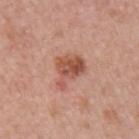follow-up — no biopsy performed (imaged during a skin exam) | anatomic site — the right upper arm | lesion diameter — ≈4 mm | automated lesion analysis — a lesion-to-skin contrast of about 8 (normalized; higher = more distinct); a nevus-likeness score of about 40/100 | lighting — white-light illumination | imaging modality — ~15 mm crop, total-body skin-cancer survey | patient — female, approximately 60 years of age.